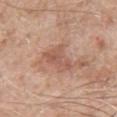workup — total-body-photography surveillance lesion; no biopsy
illumination — white-light
body site — the left upper arm
patient — male, in their 60s
automated lesion analysis — a lesion area of about 7 mm²; lesion-presence confidence of about 100/100
lesion diameter — ~4 mm (longest diameter)
image — ~15 mm tile from a whole-body skin photo